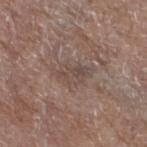Clinical impression: No biopsy was performed on this lesion — it was imaged during a full skin examination and was not determined to be concerning. Clinical summary: From the left lower leg. A 15 mm close-up tile from a total-body photography series done for melanoma screening. An algorithmic analysis of the crop reported a footprint of about 7 mm², an eccentricity of roughly 0.85, and a shape-asymmetry score of about 0.3 (0 = symmetric). The software also gave an average lesion color of about L≈46 a*≈15 b*≈21 (CIELAB) and roughly 7 lightness units darker than nearby skin. It also reported a border-irregularity index near 4/10, internal color variation of about 3.5 on a 0–10 scale, and radial color variation of about 1. A female subject aged approximately 75. Longest diameter approximately 4 mm. This is a white-light tile.